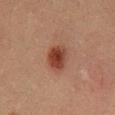Imaged during a routine full-body skin examination; the lesion was not biopsied and no histopathology is available.
A 15 mm close-up tile from a total-body photography series done for melanoma screening.
The subject is a male approximately 50 years of age.
Located on the front of the torso.